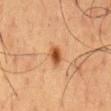The lesion was tiled from a total-body skin photograph and was not biopsied. Cropped from a whole-body photographic skin survey; the tile spans about 15 mm. Automated tile analysis of the lesion measured a border-irregularity rating of about 1.5/10, a within-lesion color-variation index near 3/10, and peripheral color asymmetry of about 1. The analysis additionally found a nevus-likeness score of about 100/100 and lesion-presence confidence of about 100/100. The subject is a male aged 58 to 62. The recorded lesion diameter is about 3 mm. From the mid back. The tile uses cross-polarized illumination.